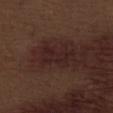biopsy status=no biopsy performed (imaged during a skin exam).